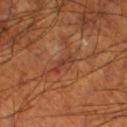biopsy status: no biopsy performed (imaged during a skin exam); anatomic site: the left lower leg; subject: male, aged approximately 70; size: about 3 mm; tile lighting: cross-polarized illumination; acquisition: ~15 mm crop, total-body skin-cancer survey.